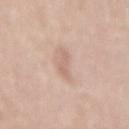notes = catalogued during a skin exam; not biopsied | site = the mid back | subject = female, aged 38 to 42 | image = total-body-photography crop, ~15 mm field of view.